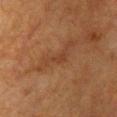Clinical impression: This lesion was catalogued during total-body skin photography and was not selected for biopsy. Clinical summary: Longest diameter approximately 3 mm. The total-body-photography lesion software estimated a footprint of about 3 mm² and two-axis asymmetry of about 0.5. It also reported border irregularity of about 5.5 on a 0–10 scale and a peripheral color-asymmetry measure near 0. The analysis additionally found an automated nevus-likeness rating near 0 out of 100. Captured under cross-polarized illumination. Cropped from a total-body skin-imaging series; the visible field is about 15 mm. Located on the chest. A female patient, aged approximately 55.